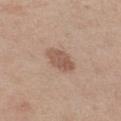The total-body-photography lesion software estimated an area of roughly 6.5 mm², a shape eccentricity near 0.75, and a shape-asymmetry score of about 0.2 (0 = symmetric). The analysis additionally found a classifier nevus-likeness of about 25/100 and a lesion-detection confidence of about 100/100. From the left thigh. A female subject in their mid-40s. A region of skin cropped from a whole-body photographic capture, roughly 15 mm wide. This is a white-light tile. Measured at roughly 3.5 mm in maximum diameter.